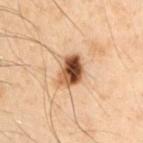biopsy status = imaged on a skin check; not biopsied | subject = male, roughly 50 years of age | lighting = cross-polarized illumination | imaging modality = ~15 mm crop, total-body skin-cancer survey | site = the left upper arm | lesion diameter = ≈3.5 mm.